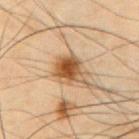Clinical impression: No biopsy was performed on this lesion — it was imaged during a full skin examination and was not determined to be concerning. Background: The lesion is located on the chest. A male subject about 55 years old. Cropped from a whole-body photographic skin survey; the tile spans about 15 mm. Captured under cross-polarized illumination. An algorithmic analysis of the crop reported a mean CIELAB color near L≈43 a*≈18 b*≈32, roughly 13 lightness units darker than nearby skin, and a normalized border contrast of about 10.5. And it measured a border-irregularity index near 3/10.